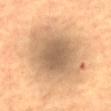No biopsy was performed on this lesion — it was imaged during a full skin examination and was not determined to be concerning.
The subject is a male approximately 65 years of age.
The lesion is located on the back.
A 15 mm crop from a total-body photograph taken for skin-cancer surveillance.